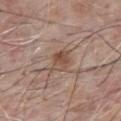Clinical impression: Part of a total-body skin-imaging series; this lesion was reviewed on a skin check and was not flagged for biopsy. Image and clinical context: A region of skin cropped from a whole-body photographic capture, roughly 15 mm wide. The lesion is on the chest. A male subject aged approximately 80.